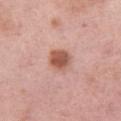| field | value |
|---|---|
| biopsy status | total-body-photography surveillance lesion; no biopsy |
| subject | female, aged approximately 50 |
| image | ~15 mm crop, total-body skin-cancer survey |
| site | the left thigh |